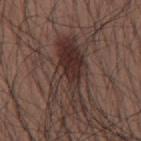{"biopsy_status": "not biopsied; imaged during a skin examination", "automated_metrics": {"cielab_L": 31, "cielab_a": 16, "cielab_b": 19, "vs_skin_darker_L": 9.0, "vs_skin_contrast_norm": 9.5, "nevus_likeness_0_100": 100, "lesion_detection_confidence_0_100": 90}, "lesion_size": {"long_diameter_mm_approx": 8.5}, "lighting": "white-light", "site": "mid back", "image": {"source": "total-body photography crop", "field_of_view_mm": 15}, "patient": {"sex": "male", "age_approx": 35}}